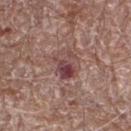Image and clinical context:
Automated tile analysis of the lesion measured an average lesion color of about L≈44 a*≈22 b*≈19 (CIELAB) and a normalized lesion–skin contrast near 8.5. And it measured a border-irregularity index near 3.5/10, internal color variation of about 10 on a 0–10 scale, and a peripheral color-asymmetry measure near 3.5. A roughly 15 mm field-of-view crop from a total-body skin photograph. The tile uses white-light illumination. Longest diameter approximately 3.5 mm. The subject is a male about 70 years old. From the right thigh.